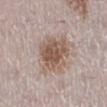follow-up — no biopsy performed (imaged during a skin exam)
size — ~5 mm (longest diameter)
subject — female, aged 48 to 52
lighting — white-light
site — the right lower leg
imaging modality — 15 mm crop, total-body photography